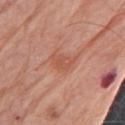notes: no biopsy performed (imaged during a skin exam)
lesion size: ≈3 mm
subject: male, in their 80s
location: the left upper arm
image: total-body-photography crop, ~15 mm field of view